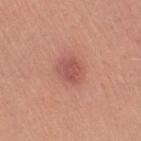workup = total-body-photography surveillance lesion; no biopsy
body site = the right thigh
patient = female, about 45 years old
tile lighting = white-light illumination
lesion size = about 3 mm
image source = 15 mm crop, total-body photography
image-analysis metrics = an area of roughly 6 mm², a shape eccentricity near 0.6, and a symmetry-axis asymmetry near 0.2; a border-irregularity rating of about 2/10, internal color variation of about 2.5 on a 0–10 scale, and peripheral color asymmetry of about 1; a nevus-likeness score of about 45/100